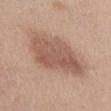Notes:
– image — total-body-photography crop, ~15 mm field of view
– location — the front of the torso
– patient — female, aged 38 to 42
– automated lesion analysis — a border-irregularity index near 3/10; an automated nevus-likeness rating near 35 out of 100 and a detector confidence of about 100 out of 100 that the crop contains a lesion
– diameter — ≈7 mm